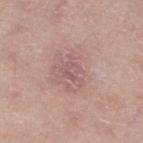| field | value |
|---|---|
| follow-up | catalogued during a skin exam; not biopsied |
| subject | female, aged around 65 |
| site | the left lower leg |
| imaging modality | ~15 mm tile from a whole-body skin photo |